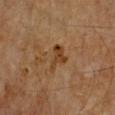Notes:
– biopsy status — catalogued during a skin exam; not biopsied
– patient — male, aged approximately 65
– TBP lesion metrics — about 8 CIELAB-L* units darker than the surrounding skin and a normalized border contrast of about 8; a border-irregularity index near 5/10 and a color-variation rating of about 3/10; a classifier nevus-likeness of about 5/100
– acquisition — total-body-photography crop, ~15 mm field of view
– lesion size — about 3.5 mm
– body site — the left lower leg
– tile lighting — cross-polarized illumination